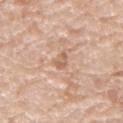Clinical impression:
Captured during whole-body skin photography for melanoma surveillance; the lesion was not biopsied.
Clinical summary:
A male patient roughly 80 years of age. This image is a 15 mm lesion crop taken from a total-body photograph. The total-body-photography lesion software estimated a lesion area of about 2.5 mm², an outline eccentricity of about 0.85 (0 = round, 1 = elongated), and a symmetry-axis asymmetry near 0.25. And it measured an average lesion color of about L≈61 a*≈20 b*≈31 (CIELAB), a lesion–skin lightness drop of about 9, and a lesion-to-skin contrast of about 6 (normalized; higher = more distinct). The software also gave a border-irregularity index near 2.5/10, a color-variation rating of about 0/10, and peripheral color asymmetry of about 0. It also reported an automated nevus-likeness rating near 0 out of 100 and a detector confidence of about 100 out of 100 that the crop contains a lesion. The lesion is on the left upper arm. About 2.5 mm across.